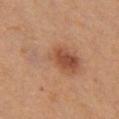Recorded during total-body skin imaging; not selected for excision or biopsy.
Measured at roughly 9 mm in maximum diameter.
Located on the chest.
A female subject aged 23–27.
A 15 mm crop from a total-body photograph taken for skin-cancer surveillance.
The total-body-photography lesion software estimated border irregularity of about 6 on a 0–10 scale, a within-lesion color-variation index near 8/10, and radial color variation of about 2.5. The software also gave a nevus-likeness score of about 95/100 and lesion-presence confidence of about 100/100.